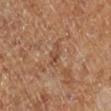automated lesion analysis: a lesion-to-skin contrast of about 5.5 (normalized; higher = more distinct); a border-irregularity rating of about 5/10 and a peripheral color-asymmetry measure near 0.5 | anatomic site: the right lower leg | lighting: cross-polarized | subject: female | lesion size: about 3 mm | acquisition: ~15 mm tile from a whole-body skin photo.